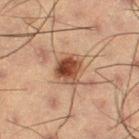Notes:
• notes: no biopsy performed (imaged during a skin exam)
• imaging modality: ~15 mm tile from a whole-body skin photo
• automated metrics: a lesion area of about 9 mm², a shape eccentricity near 0.55, and a symmetry-axis asymmetry near 0.25; a border-irregularity rating of about 2.5/10 and a within-lesion color-variation index near 8/10; an automated nevus-likeness rating near 95 out of 100 and a lesion-detection confidence of about 100/100
• diameter: about 4 mm
• patient: male, in their mid-50s
• site: the right thigh
• lighting: cross-polarized